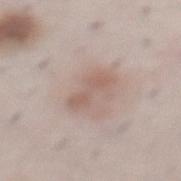| feature | finding |
|---|---|
| notes | no biopsy performed (imaged during a skin exam) |
| size | about 4 mm |
| tile lighting | white-light illumination |
| image source | ~15 mm tile from a whole-body skin photo |
| anatomic site | the abdomen |
| patient | male, roughly 40 years of age |